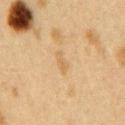Notes:
- notes: total-body-photography surveillance lesion; no biopsy
- location: the right upper arm
- image: total-body-photography crop, ~15 mm field of view
- lesion diameter: ~2.5 mm (longest diameter)
- automated lesion analysis: an average lesion color of about L≈53 a*≈14 b*≈35 (CIELAB), roughly 5 lightness units darker than nearby skin, and a normalized border contrast of about 5; a border-irregularity index near 3.5/10, internal color variation of about 0.5 on a 0–10 scale, and peripheral color asymmetry of about 0
- tile lighting: cross-polarized illumination
- patient: female, aged 38–42